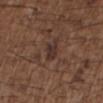Q: Was a biopsy performed?
A: no biopsy performed (imaged during a skin exam)
Q: Illumination type?
A: white-light
Q: What is the imaging modality?
A: ~15 mm tile from a whole-body skin photo
Q: What did automated image analysis measure?
A: a lesion area of about 4.5 mm², a shape eccentricity near 0.75, and two-axis asymmetry of about 0.25; a classifier nevus-likeness of about 0/100 and lesion-presence confidence of about 90/100
Q: Where on the body is the lesion?
A: the upper back
Q: What are the patient's age and sex?
A: male, roughly 50 years of age